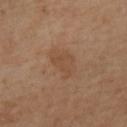This lesion was catalogued during total-body skin photography and was not selected for biopsy. A roughly 15 mm field-of-view crop from a total-body skin photograph. An algorithmic analysis of the crop reported an area of roughly 8.5 mm² and an outline eccentricity of about 0.85 (0 = round, 1 = elongated). It also reported lesion-presence confidence of about 100/100. Captured under cross-polarized illumination. A female subject aged 48 to 52. The lesion is located on the left lower leg.